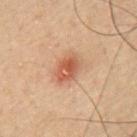Q: How was this image acquired?
A: 15 mm crop, total-body photography
Q: Who is the patient?
A: male, roughly 50 years of age
Q: Illumination type?
A: cross-polarized
Q: Where on the body is the lesion?
A: the chest